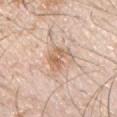Assessment:
Part of a total-body skin-imaging series; this lesion was reviewed on a skin check and was not flagged for biopsy.
Clinical summary:
From the chest. A 15 mm close-up tile from a total-body photography series done for melanoma screening. A male subject in their 30s.